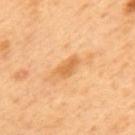Q: Is there a histopathology result?
A: no biopsy performed (imaged during a skin exam)
Q: Illumination type?
A: cross-polarized
Q: What are the patient's age and sex?
A: male, about 50 years old
Q: What is the imaging modality?
A: 15 mm crop, total-body photography
Q: Where on the body is the lesion?
A: the mid back
Q: How large is the lesion?
A: about 3 mm